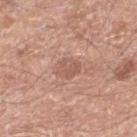{
  "biopsy_status": "not biopsied; imaged during a skin examination",
  "site": "right lower leg",
  "patient": {
    "sex": "male",
    "age_approx": 80
  },
  "lesion_size": {
    "long_diameter_mm_approx": 3.0
  },
  "image": {
    "source": "total-body photography crop",
    "field_of_view_mm": 15
  }
}